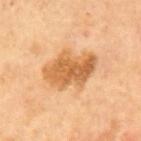Part of a total-body skin-imaging series; this lesion was reviewed on a skin check and was not flagged for biopsy. The recorded lesion diameter is about 6 mm. A 15 mm close-up tile from a total-body photography series done for melanoma screening. From the mid back. The patient is a male aged around 70.